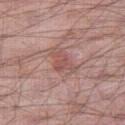Notes:
• notes · catalogued during a skin exam; not biopsied
• imaging modality · 15 mm crop, total-body photography
• lesion diameter · ≈3 mm
• site · the right thigh
• tile lighting · white-light illumination
• patient · male, about 55 years old